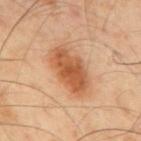Automated tile analysis of the lesion measured an area of roughly 16 mm², a shape eccentricity near 0.85, and a shape-asymmetry score of about 0.15 (0 = symmetric). And it measured a mean CIELAB color near L≈57 a*≈25 b*≈39, about 13 CIELAB-L* units darker than the surrounding skin, and a normalized lesion–skin contrast near 9. The analysis additionally found a classifier nevus-likeness of about 100/100 and a detector confidence of about 100 out of 100 that the crop contains a lesion.
Longest diameter approximately 6 mm.
A region of skin cropped from a whole-body photographic capture, roughly 15 mm wide.
A male patient approximately 40 years of age.
On the mid back.